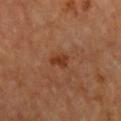notes — imaged on a skin check; not biopsied | image — 15 mm crop, total-body photography | tile lighting — cross-polarized illumination | automated metrics — a footprint of about 3 mm², a shape eccentricity near 0.75, and two-axis asymmetry of about 0.25; a nevus-likeness score of about 80/100 and a lesion-detection confidence of about 100/100 | size — about 2.5 mm | location — the right forearm | patient — female, about 40 years old.